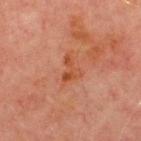{
  "biopsy_status": "not biopsied; imaged during a skin examination",
  "image": {
    "source": "total-body photography crop",
    "field_of_view_mm": 15
  },
  "site": "chest",
  "patient": {
    "sex": "male",
    "age_approx": 70
  },
  "lesion_size": {
    "long_diameter_mm_approx": 3.0
  }
}